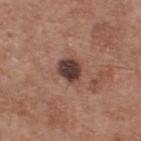Findings:
– site — the upper back
– acquisition — total-body-photography crop, ~15 mm field of view
– subject — male, approximately 55 years of age
– lighting — white-light illumination